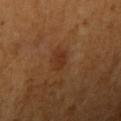Assessment:
Part of a total-body skin-imaging series; this lesion was reviewed on a skin check and was not flagged for biopsy.
Image and clinical context:
The lesion-visualizer software estimated a lesion color around L≈33 a*≈23 b*≈32 in CIELAB and a lesion-to-skin contrast of about 6.5 (normalized; higher = more distinct). The software also gave a within-lesion color-variation index near 1.5/10 and radial color variation of about 0.5. It also reported an automated nevus-likeness rating near 25 out of 100 and a lesion-detection confidence of about 100/100. The lesion is on the arm. A 15 mm close-up extracted from a 3D total-body photography capture. The subject is a female about 55 years old.